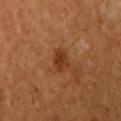Assessment: Captured during whole-body skin photography for melanoma surveillance; the lesion was not biopsied. Context: A 15 mm close-up extracted from a 3D total-body photography capture. A female patient in their mid- to late 50s. The lesion-visualizer software estimated an area of roughly 5 mm², an eccentricity of roughly 0.7, and a symmetry-axis asymmetry near 0.35. The software also gave roughly 9 lightness units darker than nearby skin and a lesion-to-skin contrast of about 8 (normalized; higher = more distinct). And it measured a nevus-likeness score of about 25/100 and lesion-presence confidence of about 100/100. Imaged with cross-polarized lighting. The lesion is located on the right upper arm.